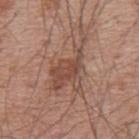Clinical impression: Recorded during total-body skin imaging; not selected for excision or biopsy. Context: Located on the mid back. Cropped from a total-body skin-imaging series; the visible field is about 15 mm. The lesion-visualizer software estimated a lesion area of about 15 mm², an eccentricity of roughly 0.85, and a shape-asymmetry score of about 0.55 (0 = symmetric). The software also gave a border-irregularity index near 9/10 and a peripheral color-asymmetry measure near 1. This is a white-light tile. The recorded lesion diameter is about 8 mm. The subject is a male in their mid- to late 50s.